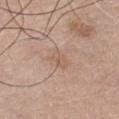<record>
<biopsy_status>not biopsied; imaged during a skin examination</biopsy_status>
<patient>
  <sex>male</sex>
  <age_approx>75</age_approx>
</patient>
<site>chest</site>
<image>
  <source>total-body photography crop</source>
  <field_of_view_mm>15</field_of_view_mm>
</image>
</record>